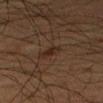{"biopsy_status": "not biopsied; imaged during a skin examination", "site": "right upper arm", "lesion_size": {"long_diameter_mm_approx": 2.5}, "image": {"source": "total-body photography crop", "field_of_view_mm": 15}, "patient": {"sex": "male", "age_approx": 60}, "lighting": "cross-polarized"}